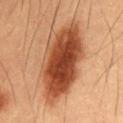Clinical impression: This lesion was catalogued during total-body skin photography and was not selected for biopsy. Background: A male patient in their mid- to late 50s. The recorded lesion diameter is about 11 mm. On the mid back. Cropped from a total-body skin-imaging series; the visible field is about 15 mm. The total-body-photography lesion software estimated a footprint of about 39 mm² and a symmetry-axis asymmetry near 0.15. The analysis additionally found a classifier nevus-likeness of about 100/100 and lesion-presence confidence of about 100/100.